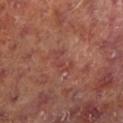Q: Was this lesion biopsied?
A: catalogued during a skin exam; not biopsied
Q: What is the lesion's diameter?
A: ~3.5 mm (longest diameter)
Q: What are the patient's age and sex?
A: male, aged 63 to 67
Q: What is the imaging modality?
A: ~15 mm crop, total-body skin-cancer survey
Q: How was the tile lit?
A: cross-polarized
Q: What is the anatomic site?
A: the left lower leg